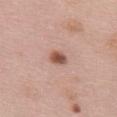| field | value |
|---|---|
| biopsy status | total-body-photography surveillance lesion; no biopsy |
| image source | ~15 mm crop, total-body skin-cancer survey |
| subject | female, aged around 60 |
| lighting | white-light |
| size | ~2.5 mm (longest diameter) |
| automated metrics | a footprint of about 4 mm² and an outline eccentricity of about 0.75 (0 = round, 1 = elongated); an average lesion color of about L≈54 a*≈23 b*≈28 (CIELAB), about 14 CIELAB-L* units darker than the surrounding skin, and a lesion-to-skin contrast of about 9.5 (normalized; higher = more distinct); border irregularity of about 2 on a 0–10 scale and a within-lesion color-variation index near 5/10; a lesion-detection confidence of about 100/100 |
| location | the back |